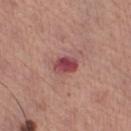Captured during whole-body skin photography for melanoma surveillance; the lesion was not biopsied.